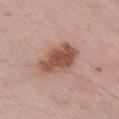The lesion was tiled from a total-body skin photograph and was not biopsied.
Imaged with white-light lighting.
A close-up tile cropped from a whole-body skin photograph, about 15 mm across.
The subject is a male aged 53 to 57.
The lesion is located on the right upper arm.